Cropped from a total-body skin-imaging series; the visible field is about 15 mm. The lesion is on the mid back. A male patient, about 40 years old: 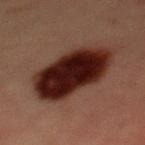| field | value |
|---|---|
| histopathologic diagnosis | a dysplastic (Clark) nevus |